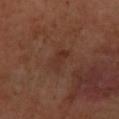Findings:
* follow-up · catalogued during a skin exam; not biopsied
* lesion size · ≈3.5 mm
* lighting · cross-polarized
* site · the right forearm
* subject · female, aged 48–52
* acquisition · 15 mm crop, total-body photography